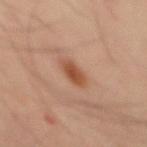Background: The lesion-visualizer software estimated a classifier nevus-likeness of about 100/100 and lesion-presence confidence of about 100/100. Captured under cross-polarized illumination. Cropped from a whole-body photographic skin survey; the tile spans about 15 mm. The recorded lesion diameter is about 3.5 mm. A male subject, aged around 50. The lesion is on the mid back.